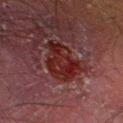Findings:
* follow-up: catalogued during a skin exam; not biopsied
* tile lighting: cross-polarized
* diameter: ≈5 mm
* imaging modality: total-body-photography crop, ~15 mm field of view
* automated lesion analysis: an area of roughly 15 mm², a shape eccentricity near 0.7, and a symmetry-axis asymmetry near 0.3
* patient: male, aged 48–52
* anatomic site: the leg